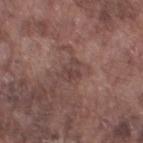Context: A 15 mm close-up tile from a total-body photography series done for melanoma screening. From the left thigh. Measured at roughly 2.5 mm in maximum diameter. Automated image analysis of the tile measured an area of roughly 3.5 mm², an eccentricity of roughly 0.8, and a symmetry-axis asymmetry near 0.4. It also reported a lesion color around L≈41 a*≈18 b*≈20 in CIELAB, roughly 7 lightness units darker than nearby skin, and a lesion-to-skin contrast of about 5.5 (normalized; higher = more distinct). The analysis additionally found border irregularity of about 4 on a 0–10 scale and a color-variation rating of about 0.5/10. A male subject aged around 75.